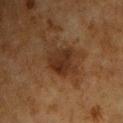Q: Is there a histopathology result?
A: catalogued during a skin exam; not biopsied
Q: What kind of image is this?
A: total-body-photography crop, ~15 mm field of view
Q: What did automated image analysis measure?
A: a footprint of about 8.5 mm², an eccentricity of roughly 0.35, and a symmetry-axis asymmetry near 0.25; a border-irregularity index near 2.5/10, internal color variation of about 2.5 on a 0–10 scale, and peripheral color asymmetry of about 1; a classifier nevus-likeness of about 25/100 and lesion-presence confidence of about 100/100
Q: Patient demographics?
A: male, aged 58 to 62
Q: What is the anatomic site?
A: the chest
Q: What is the lesion's diameter?
A: ≈3.5 mm